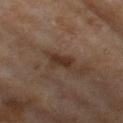Q: Was a biopsy performed?
A: imaged on a skin check; not biopsied
Q: Patient demographics?
A: female, aged 58 to 62
Q: Automated lesion metrics?
A: a lesion area of about 4.5 mm² and a shape-asymmetry score of about 0.25 (0 = symmetric); a border-irregularity rating of about 3.5/10 and a within-lesion color-variation index near 1.5/10; a classifier nevus-likeness of about 10/100 and a detector confidence of about 100 out of 100 that the crop contains a lesion
Q: What kind of image is this?
A: ~15 mm tile from a whole-body skin photo
Q: Illumination type?
A: cross-polarized illumination
Q: What is the anatomic site?
A: the left thigh
Q: What is the lesion's diameter?
A: ~3.5 mm (longest diameter)